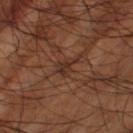Recorded during total-body skin imaging; not selected for excision or biopsy.
Imaged with cross-polarized lighting.
Measured at roughly 2.5 mm in maximum diameter.
A lesion tile, about 15 mm wide, cut from a 3D total-body photograph.
A male subject, roughly 60 years of age.
Located on the left thigh.